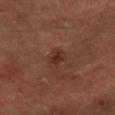Captured during whole-body skin photography for melanoma surveillance; the lesion was not biopsied. A male subject in their 40s. A 15 mm close-up extracted from a 3D total-body photography capture. The lesion's longest dimension is about 3 mm. Located on the right forearm. An algorithmic analysis of the crop reported a lesion area of about 4 mm² and a symmetry-axis asymmetry near 0.25. It also reported a nevus-likeness score of about 15/100 and a lesion-detection confidence of about 100/100.